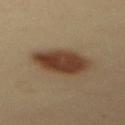Assessment: No biopsy was performed on this lesion — it was imaged during a full skin examination and was not determined to be concerning. Background: Cropped from a total-body skin-imaging series; the visible field is about 15 mm. A female patient aged 38–42. Located on the mid back.